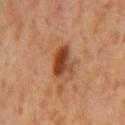Notes:
• notes: no biopsy performed (imaged during a skin exam)
• imaging modality: total-body-photography crop, ~15 mm field of view
• illumination: cross-polarized
• TBP lesion metrics: a lesion area of about 7.5 mm² and a symmetry-axis asymmetry near 0.3; an automated nevus-likeness rating near 95 out of 100 and lesion-presence confidence of about 100/100
• patient: male, in their 60s
• size: ≈3 mm
• location: the mid back